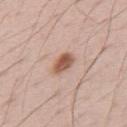workup = no biopsy performed (imaged during a skin exam)
anatomic site = the upper back
lighting = white-light
imaging modality = ~15 mm tile from a whole-body skin photo
automated lesion analysis = a nevus-likeness score of about 95/100
subject = male, about 70 years old